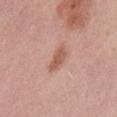No biopsy was performed on this lesion — it was imaged during a full skin examination and was not determined to be concerning. This is a white-light tile. Measured at roughly 3.5 mm in maximum diameter. A 15 mm close-up extracted from a 3D total-body photography capture. Located on the lower back. A female patient about 30 years old.